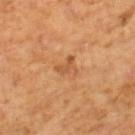Part of a total-body skin-imaging series; this lesion was reviewed on a skin check and was not flagged for biopsy.
The total-body-photography lesion software estimated an outline eccentricity of about 0.35 (0 = round, 1 = elongated) and a shape-asymmetry score of about 0.55 (0 = symmetric). And it measured an average lesion color of about L≈51 a*≈23 b*≈38 (CIELAB), a lesion–skin lightness drop of about 8, and a normalized lesion–skin contrast near 5.5. And it measured a classifier nevus-likeness of about 0/100 and a lesion-detection confidence of about 100/100.
This image is a 15 mm lesion crop taken from a total-body photograph.
This is a cross-polarized tile.
The lesion is on the right lower leg.
About 2.5 mm across.
A male patient, about 60 years old.